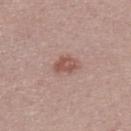biopsy status: catalogued during a skin exam; not biopsied | subject: male, about 30 years old | tile lighting: white-light illumination | lesion diameter: ≈3 mm | TBP lesion metrics: an area of roughly 5 mm² and a shape eccentricity near 0.65; a border-irregularity rating of about 2.5/10, internal color variation of about 3 on a 0–10 scale, and radial color variation of about 1; an automated nevus-likeness rating near 65 out of 100 and a detector confidence of about 100 out of 100 that the crop contains a lesion | image source: total-body-photography crop, ~15 mm field of view.